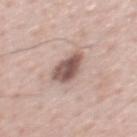Q: Was a biopsy performed?
A: catalogued during a skin exam; not biopsied
Q: What are the patient's age and sex?
A: male, roughly 60 years of age
Q: Lesion location?
A: the front of the torso
Q: Illumination type?
A: white-light illumination
Q: What is the imaging modality?
A: total-body-photography crop, ~15 mm field of view
Q: What is the lesion's diameter?
A: about 3.5 mm